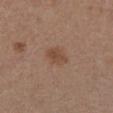Case summary:
– notes · total-body-photography surveillance lesion; no biopsy
– body site · the chest
– tile lighting · white-light
– diameter · about 3 mm
– acquisition · total-body-photography crop, ~15 mm field of view
– patient · female, roughly 30 years of age